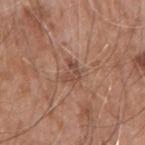Impression:
No biopsy was performed on this lesion — it was imaged during a full skin examination and was not determined to be concerning.
Background:
From the right upper arm. A 15 mm crop from a total-body photograph taken for skin-cancer surveillance. A male subject in their mid- to late 70s. Captured under white-light illumination.